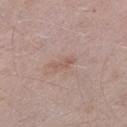Recorded during total-body skin imaging; not selected for excision or biopsy. The lesion is located on the left lower leg. This image is a 15 mm lesion crop taken from a total-body photograph. About 3 mm across. The patient is a male about 40 years old. The total-body-photography lesion software estimated an eccentricity of roughly 0.95. And it measured an average lesion color of about L≈56 a*≈18 b*≈24 (CIELAB), about 7 CIELAB-L* units darker than the surrounding skin, and a normalized border contrast of about 5.